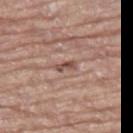Q: Is there a histopathology result?
A: no biopsy performed (imaged during a skin exam)
Q: What is the imaging modality?
A: ~15 mm tile from a whole-body skin photo
Q: Lesion location?
A: the right thigh
Q: How was the tile lit?
A: white-light
Q: Patient demographics?
A: male, roughly 80 years of age